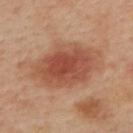notes: total-body-photography surveillance lesion; no biopsy
acquisition: ~15 mm crop, total-body skin-cancer survey
lesion diameter: ≈8 mm
subject: female, about 40 years old
illumination: cross-polarized
location: the back